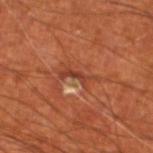Captured during whole-body skin photography for melanoma surveillance; the lesion was not biopsied.
Measured at roughly 4 mm in maximum diameter.
A close-up tile cropped from a whole-body skin photograph, about 15 mm across.
The lesion is on the left lower leg.
The tile uses cross-polarized illumination.
Automated image analysis of the tile measured a within-lesion color-variation index near 0/10 and a peripheral color-asymmetry measure near 0.
The subject is a male in their 70s.